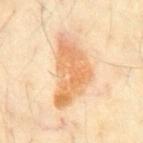Impression:
Captured during whole-body skin photography for melanoma surveillance; the lesion was not biopsied.
Image and clinical context:
A 15 mm close-up extracted from a 3D total-body photography capture. The subject is a male aged approximately 65. On the chest.